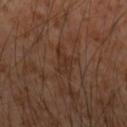Captured during whole-body skin photography for melanoma surveillance; the lesion was not biopsied.
Cropped from a whole-body photographic skin survey; the tile spans about 15 mm.
Captured under cross-polarized illumination.
The total-body-photography lesion software estimated a lesion color around L≈31 a*≈18 b*≈26 in CIELAB, about 5 CIELAB-L* units darker than the surrounding skin, and a lesion-to-skin contrast of about 5.5 (normalized; higher = more distinct). The software also gave border irregularity of about 4 on a 0–10 scale, internal color variation of about 1.5 on a 0–10 scale, and a peripheral color-asymmetry measure near 0.5. And it measured a nevus-likeness score of about 0/100 and a lesion-detection confidence of about 90/100.
Measured at roughly 3 mm in maximum diameter.
The patient is a male aged 53 to 57.
Located on the left forearm.